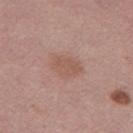Imaged during a routine full-body skin examination; the lesion was not biopsied and no histopathology is available.